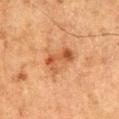<tbp_lesion>
<biopsy_status>not biopsied; imaged during a skin examination</biopsy_status>
<automated_metrics>
  <area_mm2_approx>7.5</area_mm2_approx>
  <eccentricity>0.85</eccentricity>
  <shape_asymmetry>0.3</shape_asymmetry>
  <cielab_L>45</cielab_L>
  <cielab_a>23</cielab_a>
  <cielab_b>34</cielab_b>
  <vs_skin_contrast_norm>7.0</vs_skin_contrast_norm>
  <border_irregularity_0_10>4.0</border_irregularity_0_10>
  <color_variation_0_10>6.0</color_variation_0_10>
  <peripheral_color_asymmetry>2.0</peripheral_color_asymmetry>
  <nevus_likeness_0_100>20</nevus_likeness_0_100>
  <lesion_detection_confidence_0_100>100</lesion_detection_confidence_0_100>
</automated_metrics>
<lighting>cross-polarized</lighting>
<lesion_size>
  <long_diameter_mm_approx>4.0</long_diameter_mm_approx>
</lesion_size>
<patient>
  <sex>male</sex>
  <age_approx>75</age_approx>
</patient>
<image>
  <source>total-body photography crop</source>
  <field_of_view_mm>15</field_of_view_mm>
</image>
<site>leg</site>
</tbp_lesion>